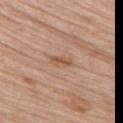Findings:
* follow-up · imaged on a skin check; not biopsied
* body site · the upper back
* diameter · ≈3 mm
* TBP lesion metrics · two-axis asymmetry of about 0.2; about 9 CIELAB-L* units darker than the surrounding skin and a normalized lesion–skin contrast near 6.5; a color-variation rating of about 0.5/10 and peripheral color asymmetry of about 0
* acquisition · 15 mm crop, total-body photography
* subject · female, approximately 65 years of age